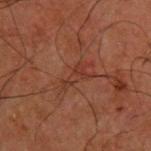notes: total-body-photography surveillance lesion; no biopsy | location: the upper back | tile lighting: cross-polarized | image-analysis metrics: an area of roughly 4 mm² and an outline eccentricity of about 0.95 (0 = round, 1 = elongated) | patient: male, aged 48 to 52 | lesion diameter: ≈3.5 mm | acquisition: 15 mm crop, total-body photography.